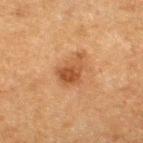Q: What lighting was used for the tile?
A: cross-polarized
Q: What did automated image analysis measure?
A: an area of roughly 7.5 mm² and a shape eccentricity near 0.75; an automated nevus-likeness rating near 80 out of 100 and lesion-presence confidence of about 100/100
Q: What are the patient's age and sex?
A: male, about 75 years old
Q: How was this image acquired?
A: 15 mm crop, total-body photography
Q: What is the lesion's diameter?
A: ~4 mm (longest diameter)
Q: Lesion location?
A: the right thigh